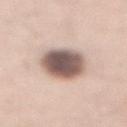Impression: Captured during whole-body skin photography for melanoma surveillance; the lesion was not biopsied. Clinical summary: On the front of the torso. This is a white-light tile. A male subject aged 58 to 62. A region of skin cropped from a whole-body photographic capture, roughly 15 mm wide. The lesion-visualizer software estimated a lesion-to-skin contrast of about 13 (normalized; higher = more distinct). Longest diameter approximately 5.5 mm.